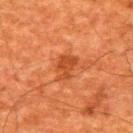This lesion was catalogued during total-body skin photography and was not selected for biopsy.
Cropped from a whole-body photographic skin survey; the tile spans about 15 mm.
From the upper back.
Imaged with cross-polarized lighting.
A male subject, aged approximately 60.
The recorded lesion diameter is about 3.5 mm.